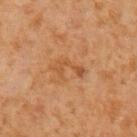notes — catalogued during a skin exam; not biopsied
image-analysis metrics — a border-irregularity index near 7.5/10 and radial color variation of about 0
body site — the left upper arm
image source — ~15 mm crop, total-body skin-cancer survey
subject — male, approximately 60 years of age
tile lighting — cross-polarized
size — ≈4 mm